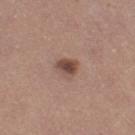workup — no biopsy performed (imaged during a skin exam) | automated lesion analysis — an area of roughly 5.5 mm², an outline eccentricity of about 0.65 (0 = round, 1 = elongated), and a symmetry-axis asymmetry near 0.25; roughly 12 lightness units darker than nearby skin; a border-irregularity index near 2.5/10, a color-variation rating of about 6/10, and peripheral color asymmetry of about 1.5 | tile lighting — white-light illumination | location — the left thigh | lesion size — ~3 mm (longest diameter) | acquisition — total-body-photography crop, ~15 mm field of view | subject — female, aged around 20.